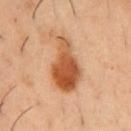Captured during whole-body skin photography for melanoma surveillance; the lesion was not biopsied.
About 7 mm across.
A male patient in their 50s.
Automated image analysis of the tile measured a shape eccentricity near 0.9 and two-axis asymmetry of about 0.4.
A 15 mm close-up tile from a total-body photography series done for melanoma screening.
On the chest.